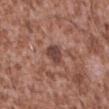{"biopsy_status": "not biopsied; imaged during a skin examination", "patient": {"sex": "male", "age_approx": 45}, "lighting": "white-light", "automated_metrics": {"area_mm2_approx": 5.0, "nevus_likeness_0_100": 0, "lesion_detection_confidence_0_100": 100}, "image": {"source": "total-body photography crop", "field_of_view_mm": 15}, "site": "abdomen"}